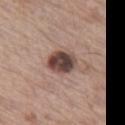- workup: no biopsy performed (imaged during a skin exam)
- image source: ~15 mm tile from a whole-body skin photo
- size: about 3.5 mm
- body site: the left thigh
- image-analysis metrics: an area of roughly 9 mm², an eccentricity of roughly 0.6, and two-axis asymmetry of about 0.15; a border-irregularity index near 1.5/10, a color-variation rating of about 6.5/10, and peripheral color asymmetry of about 2.5
- subject: male, aged 63 to 67